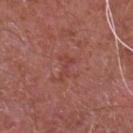notes: total-body-photography surveillance lesion; no biopsy
anatomic site: the chest
imaging modality: ~15 mm tile from a whole-body skin photo
patient: male, roughly 65 years of age